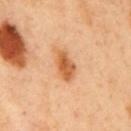{
  "biopsy_status": "not biopsied; imaged during a skin examination",
  "image": {
    "source": "total-body photography crop",
    "field_of_view_mm": 15
  },
  "patient": {
    "sex": "male",
    "age_approx": 50
  },
  "site": "mid back"
}